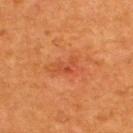Impression:
Recorded during total-body skin imaging; not selected for excision or biopsy.
Background:
Measured at roughly 3 mm in maximum diameter. A 15 mm close-up tile from a total-body photography series done for melanoma screening. A male patient, about 55 years old. The lesion is on the upper back. This is a cross-polarized tile.